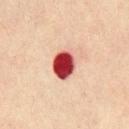Clinical impression: This lesion was catalogued during total-body skin photography and was not selected for biopsy. Context: Located on the front of the torso. The subject is a male about 45 years old. Cropped from a whole-body photographic skin survey; the tile spans about 15 mm. This is a cross-polarized tile.